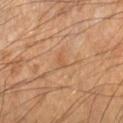{"lesion_size": {"long_diameter_mm_approx": 2.0}, "site": "left forearm", "image": {"source": "total-body photography crop", "field_of_view_mm": 15}, "patient": {"sex": "male", "age_approx": 60}, "lighting": "cross-polarized", "automated_metrics": {"eccentricity": 0.75, "shape_asymmetry": 0.4, "vs_skin_darker_L": 5.0, "vs_skin_contrast_norm": 4.5, "color_variation_0_10": 0.5, "peripheral_color_asymmetry": 0.0}}